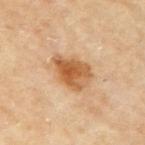site: the right upper arm | subject: female, aged around 60 | image source: ~15 mm crop, total-body skin-cancer survey | lesion size: ~5 mm (longest diameter).